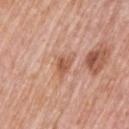<tbp_lesion>
  <patient>
    <sex>male</sex>
    <age_approx>75</age_approx>
  </patient>
  <image>
    <source>total-body photography crop</source>
    <field_of_view_mm>15</field_of_view_mm>
  </image>
  <site>left upper arm</site>
</tbp_lesion>